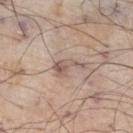follow-up: imaged on a skin check; not biopsied | patient: male, roughly 70 years of age | image source: total-body-photography crop, ~15 mm field of view | lesion diameter: about 3.5 mm | tile lighting: white-light | site: the leg.